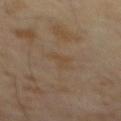| field | value |
|---|---|
| workup | total-body-photography surveillance lesion; no biopsy |
| patient | male, aged 63–67 |
| lesion size | about 2.5 mm |
| lighting | cross-polarized |
| acquisition | ~15 mm tile from a whole-body skin photo |